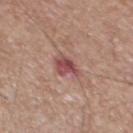Captured during whole-body skin photography for melanoma surveillance; the lesion was not biopsied. On the chest. A 15 mm close-up extracted from a 3D total-body photography capture. A male patient, in their mid- to late 60s.